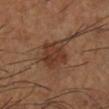Q: Was a biopsy performed?
A: imaged on a skin check; not biopsied
Q: How was this image acquired?
A: 15 mm crop, total-body photography
Q: What did automated image analysis measure?
A: a lesion–skin lightness drop of about 9 and a normalized lesion–skin contrast near 8; a border-irregularity index near 4.5/10 and a within-lesion color-variation index near 4/10
Q: Patient demographics?
A: male, roughly 65 years of age
Q: Where on the body is the lesion?
A: the left lower leg
Q: How large is the lesion?
A: ≈5.5 mm